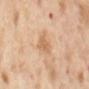Assessment:
The lesion was photographed on a routine skin check and not biopsied; there is no pathology result.
Image and clinical context:
This image is a 15 mm lesion crop taken from a total-body photograph. Captured under cross-polarized illumination. A female patient aged 53–57. From the back.